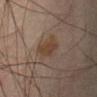The lesion was tiled from a total-body skin photograph and was not biopsied. A male patient roughly 65 years of age. The lesion is located on the abdomen. Longest diameter approximately 3.5 mm. Automated image analysis of the tile measured a lesion area of about 8 mm², an eccentricity of roughly 0.75, and a shape-asymmetry score of about 0.3 (0 = symmetric). It also reported a lesion color around L≈34 a*≈13 b*≈23 in CIELAB and about 6 CIELAB-L* units darker than the surrounding skin. It also reported a border-irregularity rating of about 3.5/10 and a within-lesion color-variation index near 2.5/10. The software also gave an automated nevus-likeness rating near 30 out of 100 and a lesion-detection confidence of about 100/100. A region of skin cropped from a whole-body photographic capture, roughly 15 mm wide. Captured under cross-polarized illumination.